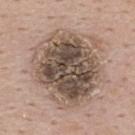workup: catalogued during a skin exam; not biopsied | illumination: white-light | location: the upper back | subject: male, in their mid- to late 50s | imaging modality: 15 mm crop, total-body photography.